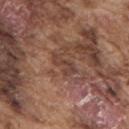Clinical impression:
This lesion was catalogued during total-body skin photography and was not selected for biopsy.
Image and clinical context:
A 15 mm close-up tile from a total-body photography series done for melanoma screening. The lesion's longest dimension is about 3.5 mm. A male patient, aged approximately 75. Automated image analysis of the tile measured a lesion color around L≈42 a*≈19 b*≈25 in CIELAB, roughly 7 lightness units darker than nearby skin, and a normalized border contrast of about 6. And it measured a border-irregularity index near 3.5/10, a within-lesion color-variation index near 2/10, and peripheral color asymmetry of about 0.5. From the upper back.